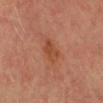The lesion was tiled from a total-body skin photograph and was not biopsied.
The subject is a male aged approximately 70.
A 15 mm crop from a total-body photograph taken for skin-cancer surveillance.
Imaged with cross-polarized lighting.
The lesion's longest dimension is about 3.5 mm.
The lesion is on the head or neck.
An algorithmic analysis of the crop reported a shape eccentricity near 0.8 and two-axis asymmetry of about 0.25.